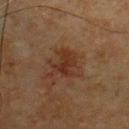Assessment:
The lesion was tiled from a total-body skin photograph and was not biopsied.
Background:
About 4 mm across. A 15 mm close-up tile from a total-body photography series done for melanoma screening. The patient is a male about 65 years old. The lesion is on the chest. This is a cross-polarized tile.